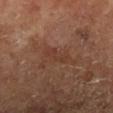Part of a total-body skin-imaging series; this lesion was reviewed on a skin check and was not flagged for biopsy. The total-body-photography lesion software estimated a lesion area of about 3.5 mm², a shape eccentricity near 0.9, and two-axis asymmetry of about 0.5. The analysis additionally found a mean CIELAB color near L≈37 a*≈21 b*≈27, roughly 5 lightness units darker than nearby skin, and a normalized border contrast of about 5. The software also gave a border-irregularity index near 6/10 and radial color variation of about 0. It also reported a classifier nevus-likeness of about 0/100. A male subject, aged 63–67. Measured at roughly 3.5 mm in maximum diameter. A region of skin cropped from a whole-body photographic capture, roughly 15 mm wide. On the right lower leg. The tile uses cross-polarized illumination.